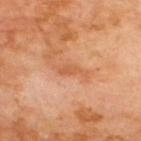| field | value |
|---|---|
| notes | total-body-photography surveillance lesion; no biopsy |
| subject | male, approximately 70 years of age |
| site | the upper back |
| image | total-body-photography crop, ~15 mm field of view |
| image-analysis metrics | an outline eccentricity of about 0.9 (0 = round, 1 = elongated) and a shape-asymmetry score of about 0.5 (0 = symmetric); a lesion color around L≈57 a*≈26 b*≈39 in CIELAB, roughly 7 lightness units darker than nearby skin, and a normalized lesion–skin contrast near 5.5; border irregularity of about 5.5 on a 0–10 scale, a color-variation rating of about 1/10, and a peripheral color-asymmetry measure near 0; a detector confidence of about 100 out of 100 that the crop contains a lesion |
| size | about 3.5 mm |
| tile lighting | cross-polarized |